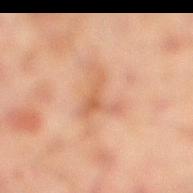follow-up=imaged on a skin check; not biopsied
body site=the right lower leg
patient=male, in their mid- to late 40s
image source=~15 mm crop, total-body skin-cancer survey
lesion size=~4 mm (longest diameter)